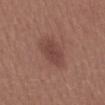Q: Is there a histopathology result?
A: catalogued during a skin exam; not biopsied
Q: Where on the body is the lesion?
A: the back
Q: Illumination type?
A: white-light
Q: How was this image acquired?
A: 15 mm crop, total-body photography
Q: Patient demographics?
A: female, about 25 years old
Q: Lesion size?
A: about 4 mm